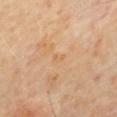Imaged during a routine full-body skin examination; the lesion was not biopsied and no histopathology is available. A roughly 15 mm field-of-view crop from a total-body skin photograph. A male subject roughly 65 years of age. Located on the mid back.